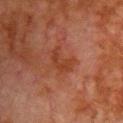This lesion was catalogued during total-body skin photography and was not selected for biopsy. From the chest. This is a cross-polarized tile. Cropped from a whole-body photographic skin survey; the tile spans about 15 mm. A male patient, aged 78 to 82.